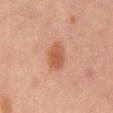Assessment:
The lesion was tiled from a total-body skin photograph and was not biopsied.
Acquisition and patient details:
About 3.5 mm across. Captured under cross-polarized illumination. A roughly 15 mm field-of-view crop from a total-body skin photograph. The lesion is on the mid back. A male patient, aged approximately 65. Automated image analysis of the tile measured a lesion area of about 6.5 mm² and an eccentricity of roughly 0.75. And it measured a border-irregularity rating of about 2.5/10 and a peripheral color-asymmetry measure near 1.